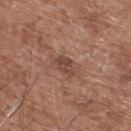<case>
<biopsy_status>not biopsied; imaged during a skin examination</biopsy_status>
<site>back</site>
<automated_metrics>
  <vs_skin_darker_L>9.0</vs_skin_darker_L>
  <vs_skin_contrast_norm>7.0</vs_skin_contrast_norm>
  <border_irregularity_0_10>3.0</border_irregularity_0_10>
  <color_variation_0_10>3.0</color_variation_0_10>
  <peripheral_color_asymmetry>1.5</peripheral_color_asymmetry>
</automated_metrics>
<lighting>white-light</lighting>
<patient>
  <sex>male</sex>
  <age_approx>75</age_approx>
</patient>
<lesion_size>
  <long_diameter_mm_approx>3.5</long_diameter_mm_approx>
</lesion_size>
<image>
  <source>total-body photography crop</source>
  <field_of_view_mm>15</field_of_view_mm>
</image>
</case>